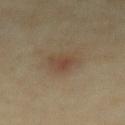Recorded during total-body skin imaging; not selected for excision or biopsy. Captured under cross-polarized illumination. A male subject aged approximately 70. From the abdomen. Longest diameter approximately 3.5 mm. A lesion tile, about 15 mm wide, cut from a 3D total-body photograph.